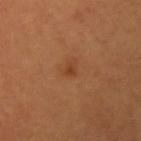Assessment:
The lesion was tiled from a total-body skin photograph and was not biopsied.
Background:
Automated image analysis of the tile measured about 7 CIELAB-L* units darker than the surrounding skin and a normalized lesion–skin contrast near 6. The analysis additionally found a border-irregularity index near 3.5/10. A male patient, in their mid- to late 60s. The lesion is on the right upper arm. Cropped from a total-body skin-imaging series; the visible field is about 15 mm. The tile uses cross-polarized illumination. Approximately 1.5 mm at its widest.